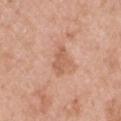<case>
  <biopsy_status>not biopsied; imaged during a skin examination</biopsy_status>
  <automated_metrics>
    <area_mm2_approx>5.0</area_mm2_approx>
    <eccentricity>0.75</eccentricity>
    <shape_asymmetry>0.35</shape_asymmetry>
    <vs_skin_darker_L>8.0</vs_skin_darker_L>
    <nevus_likeness_0_100>0</nevus_likeness_0_100>
    <lesion_detection_confidence_0_100>100</lesion_detection_confidence_0_100>
  </automated_metrics>
  <image>
    <source>total-body photography crop</source>
    <field_of_view_mm>15</field_of_view_mm>
  </image>
  <lesion_size>
    <long_diameter_mm_approx>3.5</long_diameter_mm_approx>
  </lesion_size>
  <lighting>white-light</lighting>
  <site>left upper arm</site>
  <patient>
    <sex>male</sex>
    <age_approx>50</age_approx>
  </patient>
</case>